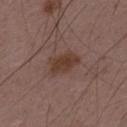Findings:
- workup: catalogued during a skin exam; not biopsied
- subject: male, aged 48 to 52
- diameter: ≈4 mm
- image: 15 mm crop, total-body photography
- body site: the back
- lighting: white-light